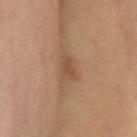biopsy status — total-body-photography surveillance lesion; no biopsy